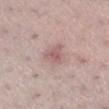No biopsy was performed on this lesion — it was imaged during a full skin examination and was not determined to be concerning. The lesion is located on the leg. A female patient in their mid-30s. A 15 mm close-up extracted from a 3D total-body photography capture.